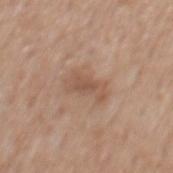Clinical impression: Recorded during total-body skin imaging; not selected for excision or biopsy.